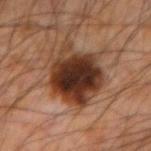Image and clinical context:
Located on the left forearm. About 6.5 mm across. Automated tile analysis of the lesion measured a symmetry-axis asymmetry near 0.2. And it measured a border-irregularity rating of about 3/10, internal color variation of about 8 on a 0–10 scale, and radial color variation of about 2. The software also gave an automated nevus-likeness rating near 70 out of 100 and lesion-presence confidence of about 100/100. Cropped from a total-body skin-imaging series; the visible field is about 15 mm. A male patient in their mid-60s. Imaged with cross-polarized lighting.
Conclusion:
Histopathologically confirmed as a seborrheic keratosis (benign).